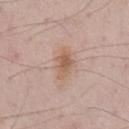| feature | finding |
|---|---|
| notes | imaged on a skin check; not biopsied |
| illumination | white-light |
| lesion size | ~4 mm (longest diameter) |
| subject | male, roughly 70 years of age |
| location | the front of the torso |
| acquisition | ~15 mm crop, total-body skin-cancer survey |